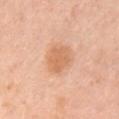Clinical impression: No biopsy was performed on this lesion — it was imaged during a full skin examination and was not determined to be concerning. Acquisition and patient details: The patient is a female about 30 years old. A lesion tile, about 15 mm wide, cut from a 3D total-body photograph. The lesion is located on the chest.